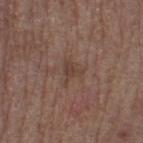No biopsy was performed on this lesion — it was imaged during a full skin examination and was not determined to be concerning. A female subject aged approximately 65. From the left thigh. This image is a 15 mm lesion crop taken from a total-body photograph. Measured at roughly 3 mm in maximum diameter.